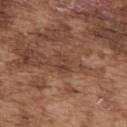Recorded during total-body skin imaging; not selected for excision or biopsy. A roughly 15 mm field-of-view crop from a total-body skin photograph. The lesion is located on the upper back. The tile uses white-light illumination. A male patient, aged 73–77.